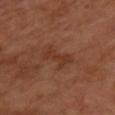This lesion was catalogued during total-body skin photography and was not selected for biopsy. The subject is a male roughly 65 years of age. This image is a 15 mm lesion crop taken from a total-body photograph. An algorithmic analysis of the crop reported a lesion area of about 6 mm² and a symmetry-axis asymmetry near 0.3. And it measured a border-irregularity rating of about 4.5/10, a within-lesion color-variation index near 2/10, and radial color variation of about 0.5. The tile uses cross-polarized illumination. The recorded lesion diameter is about 4 mm.